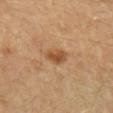notes = no biopsy performed (imaged during a skin exam)
size = about 3 mm
imaging modality = ~15 mm tile from a whole-body skin photo
subject = female, aged around 40
image-analysis metrics = an average lesion color of about L≈52 a*≈22 b*≈38 (CIELAB), a lesion–skin lightness drop of about 11, and a normalized border contrast of about 7.5; a peripheral color-asymmetry measure near 1; an automated nevus-likeness rating near 80 out of 100 and lesion-presence confidence of about 100/100
anatomic site = the arm
lighting = cross-polarized illumination